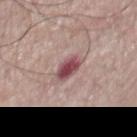Clinical impression: Captured during whole-body skin photography for melanoma surveillance; the lesion was not biopsied. Background: A 15 mm close-up tile from a total-body photography series done for melanoma screening. A male subject in their mid-60s. The lesion-visualizer software estimated an area of roughly 6 mm² and an outline eccentricity of about 0.75 (0 = round, 1 = elongated). The software also gave a lesion–skin lightness drop of about 15 and a lesion-to-skin contrast of about 10.5 (normalized; higher = more distinct). About 3 mm across. The lesion is located on the chest. The tile uses white-light illumination.